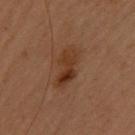follow-up = catalogued during a skin exam; not biopsied | subject = female, aged around 40 | image = total-body-photography crop, ~15 mm field of view | anatomic site = the upper back | lesion diameter = about 5 mm | tile lighting = cross-polarized.